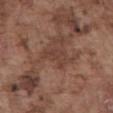Image and clinical context:
Automated tile analysis of the lesion measured a footprint of about 5.5 mm², a shape eccentricity near 0.75, and a shape-asymmetry score of about 0.6 (0 = symmetric). The analysis additionally found a lesion color around L≈40 a*≈19 b*≈24 in CIELAB, a lesion–skin lightness drop of about 6, and a lesion-to-skin contrast of about 5.5 (normalized; higher = more distinct). Cropped from a whole-body photographic skin survey; the tile spans about 15 mm. Measured at roughly 3.5 mm in maximum diameter. The subject is a male aged around 75. Imaged with white-light lighting. From the abdomen.